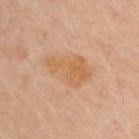Clinical impression: This lesion was catalogued during total-body skin photography and was not selected for biopsy. Background: From the upper back. Imaged with cross-polarized lighting. A close-up tile cropped from a whole-body skin photograph, about 15 mm across. The patient is a male roughly 60 years of age.